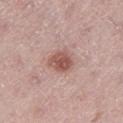Captured during whole-body skin photography for melanoma surveillance; the lesion was not biopsied. Located on the left thigh. A male patient aged around 65. Approximately 3.5 mm at its widest. Captured under white-light illumination. Cropped from a total-body skin-imaging series; the visible field is about 15 mm.